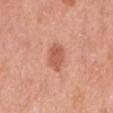workup — catalogued during a skin exam; not biopsied
lesion size — ≈3.5 mm
site — the mid back
imaging modality — ~15 mm tile from a whole-body skin photo
patient — male, aged 68–72